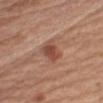– follow-up — no biopsy performed (imaged during a skin exam)